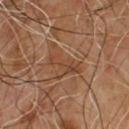No biopsy was performed on this lesion — it was imaged during a full skin examination and was not determined to be concerning. The patient is a male aged approximately 65. A close-up tile cropped from a whole-body skin photograph, about 15 mm across.